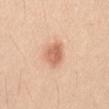The lesion was photographed on a routine skin check and not biopsied; there is no pathology result. The patient is a male aged 23–27. Automated tile analysis of the lesion measured a border-irregularity index near 2/10, a color-variation rating of about 3.5/10, and peripheral color asymmetry of about 1. The analysis additionally found an automated nevus-likeness rating near 100 out of 100 and a detector confidence of about 100 out of 100 that the crop contains a lesion. The lesion is on the lower back. The lesion's longest dimension is about 3.5 mm. A close-up tile cropped from a whole-body skin photograph, about 15 mm across. This is a white-light tile.